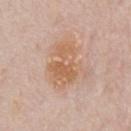Notes:
• workup: imaged on a skin check; not biopsied
• subject: male, in their mid- to late 70s
• TBP lesion metrics: a nevus-likeness score of about 5/100 and lesion-presence confidence of about 100/100
• lesion diameter: ~5.5 mm (longest diameter)
• tile lighting: white-light illumination
• location: the chest
• acquisition: ~15 mm tile from a whole-body skin photo